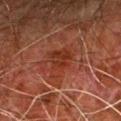No biopsy was performed on this lesion — it was imaged during a full skin examination and was not determined to be concerning. Imaged with cross-polarized lighting. Located on the arm. The subject is a male aged 78 to 82. A region of skin cropped from a whole-body photographic capture, roughly 15 mm wide. The recorded lesion diameter is about 4.5 mm.